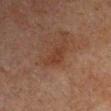Clinical impression:
This lesion was catalogued during total-body skin photography and was not selected for biopsy.